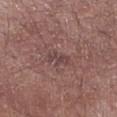Captured during whole-body skin photography for melanoma surveillance; the lesion was not biopsied.
A roughly 15 mm field-of-view crop from a total-body skin photograph.
The lesion is on the right lower leg.
Captured under white-light illumination.
A male patient about 55 years old.
Automated image analysis of the tile measured a footprint of about 3.5 mm² and a shape-asymmetry score of about 0.4 (0 = symmetric). The software also gave roughly 7 lightness units darker than nearby skin. It also reported a nevus-likeness score of about 0/100 and a detector confidence of about 95 out of 100 that the crop contains a lesion.